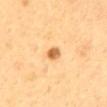Part of a total-body skin-imaging series; this lesion was reviewed on a skin check and was not flagged for biopsy. On the back. A female subject aged 48 to 52. A roughly 15 mm field-of-view crop from a total-body skin photograph. An algorithmic analysis of the crop reported an area of roughly 3 mm² and an eccentricity of roughly 0.65. The analysis additionally found an average lesion color of about L≈65 a*≈23 b*≈45 (CIELAB), about 15 CIELAB-L* units darker than the surrounding skin, and a lesion-to-skin contrast of about 9 (normalized; higher = more distinct). The analysis additionally found a border-irregularity rating of about 1.5/10, a color-variation rating of about 3/10, and radial color variation of about 1. And it measured an automated nevus-likeness rating near 95 out of 100. Longest diameter approximately 2 mm. This is a cross-polarized tile.